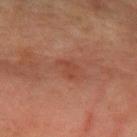This lesion was catalogued during total-body skin photography and was not selected for biopsy. From the left forearm. This image is a 15 mm lesion crop taken from a total-body photograph. The subject is a female roughly 55 years of age. The lesion's longest dimension is about 2.5 mm. The lesion-visualizer software estimated a mean CIELAB color near L≈38 a*≈23 b*≈27, about 5 CIELAB-L* units darker than the surrounding skin, and a lesion-to-skin contrast of about 5 (normalized; higher = more distinct). The analysis additionally found a border-irregularity index near 5/10 and peripheral color asymmetry of about 0.5. This is a cross-polarized tile.